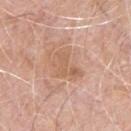The lesion was photographed on a routine skin check and not biopsied; there is no pathology result. A male subject aged approximately 70. An algorithmic analysis of the crop reported border irregularity of about 5.5 on a 0–10 scale, a color-variation rating of about 2.5/10, and peripheral color asymmetry of about 1. The software also gave an automated nevus-likeness rating near 0 out of 100 and a lesion-detection confidence of about 100/100. The lesion is on the chest. This is a white-light tile. The recorded lesion diameter is about 4 mm. A close-up tile cropped from a whole-body skin photograph, about 15 mm across.